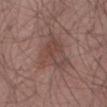automated lesion analysis: a lesion area of about 16 mm² and a shape-asymmetry score of about 0.45 (0 = symmetric); a mean CIELAB color near L≈45 a*≈19 b*≈22, about 7 CIELAB-L* units darker than the surrounding skin, and a normalized border contrast of about 5.5; border irregularity of about 4.5 on a 0–10 scale, a within-lesion color-variation index near 4/10, and peripheral color asymmetry of about 1; a classifier nevus-likeness of about 0/100 and a lesion-detection confidence of about 100/100
site: the right thigh
lesion size: ≈5.5 mm
illumination: white-light
image source: ~15 mm crop, total-body skin-cancer survey
patient: male, aged around 55